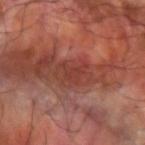Impression:
No biopsy was performed on this lesion — it was imaged during a full skin examination and was not determined to be concerning.
Image and clinical context:
The lesion is located on the arm. A lesion tile, about 15 mm wide, cut from a 3D total-body photograph. About 3.5 mm across. Automated image analysis of the tile measured a lesion color around L≈38 a*≈28 b*≈27 in CIELAB, roughly 7 lightness units darker than nearby skin, and a lesion-to-skin contrast of about 6 (normalized; higher = more distinct). And it measured border irregularity of about 7.5 on a 0–10 scale and radial color variation of about 0.5. It also reported a nevus-likeness score of about 0/100 and lesion-presence confidence of about 75/100. A male patient, aged approximately 60.